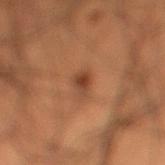{"biopsy_status": "not biopsied; imaged during a skin examination", "site": "right lower leg", "lighting": "cross-polarized", "image": {"source": "total-body photography crop", "field_of_view_mm": 15}, "lesion_size": {"long_diameter_mm_approx": 2.5}, "patient": {"sex": "male", "age_approx": 50}}